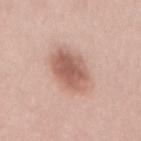Approximately 6 mm at its widest. A female patient, in their mid-20s. Automated image analysis of the tile measured an area of roughly 16 mm², an eccentricity of roughly 0.85, and a symmetry-axis asymmetry near 0.15. The software also gave a border-irregularity rating of about 2/10 and peripheral color asymmetry of about 1.5. And it measured a classifier nevus-likeness of about 95/100 and a lesion-detection confidence of about 100/100. Captured under white-light illumination. This image is a 15 mm lesion crop taken from a total-body photograph. The lesion is located on the mid back.